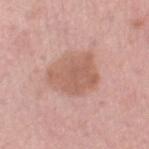Assessment: Recorded during total-body skin imaging; not selected for excision or biopsy. Context: Cropped from a total-body skin-imaging series; the visible field is about 15 mm. A female patient, about 55 years old. From the right thigh.